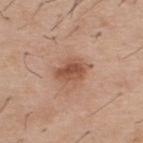site=the upper back; imaging modality=total-body-photography crop, ~15 mm field of view; illumination=white-light; TBP lesion metrics=a border-irregularity rating of about 2/10, a within-lesion color-variation index near 4/10, and a peripheral color-asymmetry measure near 1; diameter=~3.5 mm (longest diameter); subject=male, approximately 40 years of age.